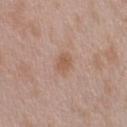The lesion was tiled from a total-body skin photograph and was not biopsied. About 3 mm across. The subject is a male roughly 45 years of age. This image is a 15 mm lesion crop taken from a total-body photograph. The lesion is on the arm.